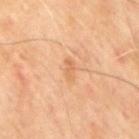| field | value |
|---|---|
| workup | total-body-photography surveillance lesion; no biopsy |
| anatomic site | the mid back |
| tile lighting | cross-polarized |
| lesion diameter | ~3 mm (longest diameter) |
| imaging modality | ~15 mm tile from a whole-body skin photo |
| subject | male, about 65 years old |